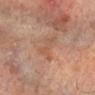workup: total-body-photography surveillance lesion; no biopsy | image: 15 mm crop, total-body photography | subject: male, about 65 years old | body site: the arm | automated metrics: a mean CIELAB color near L≈48 a*≈19 b*≈28, a lesion–skin lightness drop of about 5, and a lesion-to-skin contrast of about 5 (normalized; higher = more distinct); a border-irregularity rating of about 7/10, internal color variation of about 3.5 on a 0–10 scale, and a peripheral color-asymmetry measure near 1.